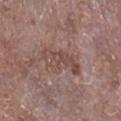Recorded during total-body skin imaging; not selected for excision or biopsy.
The patient is a female aged 73 to 77.
A lesion tile, about 15 mm wide, cut from a 3D total-body photograph.
Automated image analysis of the tile measured a footprint of about 9 mm² and an outline eccentricity of about 0.9 (0 = round, 1 = elongated). The analysis additionally found a border-irregularity index near 6/10 and a peripheral color-asymmetry measure near 1. It also reported a nevus-likeness score of about 0/100 and a detector confidence of about 65 out of 100 that the crop contains a lesion.
Imaged with white-light lighting.
Measured at roughly 5.5 mm in maximum diameter.
On the leg.